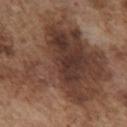notes = no biopsy performed (imaged during a skin exam); location = the chest; image = total-body-photography crop, ~15 mm field of view; subject = male, aged approximately 75.